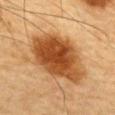No biopsy was performed on this lesion — it was imaged during a full skin examination and was not determined to be concerning. A male patient, approximately 85 years of age or older. This image is a 15 mm lesion crop taken from a total-body photograph. Automated image analysis of the tile measured a color-variation rating of about 5.5/10 and a peripheral color-asymmetry measure near 1.5. About 8.5 mm across. On the chest.